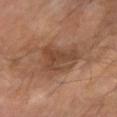Located on the right forearm. A lesion tile, about 15 mm wide, cut from a 3D total-body photograph. An algorithmic analysis of the crop reported an average lesion color of about L≈41 a*≈18 b*≈28 (CIELAB), a lesion–skin lightness drop of about 8, and a normalized lesion–skin contrast near 6.5. The analysis additionally found a border-irregularity rating of about 6.5/10, internal color variation of about 2.5 on a 0–10 scale, and radial color variation of about 1. And it measured a nevus-likeness score of about 5/100. Measured at roughly 5 mm in maximum diameter. The patient is a male aged around 65. The tile uses cross-polarized illumination.